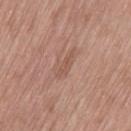| field | value |
|---|---|
| biopsy status | catalogued during a skin exam; not biopsied |
| patient | female, in their mid- to late 70s |
| body site | the left thigh |
| acquisition | total-body-photography crop, ~15 mm field of view |
| lighting | white-light illumination |